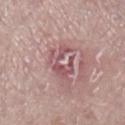Impression:
Part of a total-body skin-imaging series; this lesion was reviewed on a skin check and was not flagged for biopsy.
Acquisition and patient details:
A female subject, approximately 70 years of age. This image is a 15 mm lesion crop taken from a total-body photograph. Captured under white-light illumination. Automated tile analysis of the lesion measured a lesion area of about 8 mm². It also reported a mean CIELAB color near L≈53 a*≈25 b*≈17 and a normalized border contrast of about 7. It also reported a border-irregularity rating of about 8.5/10 and a color-variation rating of about 1.5/10. And it measured a classifier nevus-likeness of about 0/100 and a detector confidence of about 50 out of 100 that the crop contains a lesion. Located on the leg.